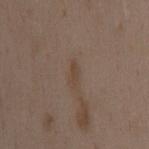biopsy_status: not biopsied; imaged during a skin examination
lesion_size:
  long_diameter_mm_approx: 3.0
site: mid back
image:
  source: total-body photography crop
  field_of_view_mm: 15
lighting: white-light
patient:
  sex: female
  age_approx: 35
automated_metrics:
  area_mm2_approx: 2.5
  eccentricity: 0.95
  shape_asymmetry: 0.4
  cielab_L: 43
  cielab_a: 13
  cielab_b: 25
  vs_skin_contrast_norm: 5.5
  border_irregularity_0_10: 4.0
  peripheral_color_asymmetry: 0.0